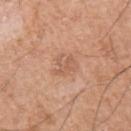notes = no biopsy performed (imaged during a skin exam)
image source = 15 mm crop, total-body photography
patient = male, in their mid- to late 50s
tile lighting = white-light
automated lesion analysis = an area of roughly 5.5 mm²; a color-variation rating of about 3/10 and peripheral color asymmetry of about 1
body site = the left upper arm